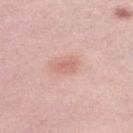This lesion was catalogued during total-body skin photography and was not selected for biopsy. A region of skin cropped from a whole-body photographic capture, roughly 15 mm wide. The tile uses white-light illumination. From the left lower leg. A female subject, aged 63–67.